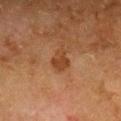Impression: This lesion was catalogued during total-body skin photography and was not selected for biopsy. Context: The lesion is located on the chest. An algorithmic analysis of the crop reported an outline eccentricity of about 0.55 (0 = round, 1 = elongated) and a shape-asymmetry score of about 0.35 (0 = symmetric). It also reported a classifier nevus-likeness of about 15/100 and lesion-presence confidence of about 100/100. This image is a 15 mm lesion crop taken from a total-body photograph. About 2.5 mm across. The patient is a female about 50 years old. This is a cross-polarized tile.